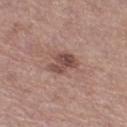Assessment:
No biopsy was performed on this lesion — it was imaged during a full skin examination and was not determined to be concerning.
Acquisition and patient details:
Imaged with white-light lighting. Measured at roughly 3.5 mm in maximum diameter. A female subject aged 38 to 42. A 15 mm close-up extracted from a 3D total-body photography capture. The lesion is on the right thigh. Automated tile analysis of the lesion measured an area of roughly 7 mm², an outline eccentricity of about 0.65 (0 = round, 1 = elongated), and a shape-asymmetry score of about 0.3 (0 = symmetric). The software also gave border irregularity of about 3 on a 0–10 scale and a color-variation rating of about 5/10.